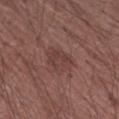No biopsy was performed on this lesion — it was imaged during a full skin examination and was not determined to be concerning.
From the right upper arm.
A roughly 15 mm field-of-view crop from a total-body skin photograph.
A male patient roughly 65 years of age.
Longest diameter approximately 4 mm.
This is a white-light tile.